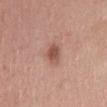follow-up — total-body-photography surveillance lesion; no biopsy | illumination — white-light | image — 15 mm crop, total-body photography | subject — female, about 55 years old | location — the leg | automated lesion analysis — a within-lesion color-variation index near 2.5/10 and radial color variation of about 0.5; a classifier nevus-likeness of about 90/100 and a detector confidence of about 100 out of 100 that the crop contains a lesion | diameter — ~4 mm (longest diameter).